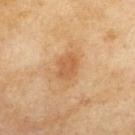Captured during whole-body skin photography for melanoma surveillance; the lesion was not biopsied.
A female subject in their 60s.
The lesion is located on the back.
Automated image analysis of the tile measured an area of roughly 6.5 mm², an outline eccentricity of about 0.65 (0 = round, 1 = elongated), and a shape-asymmetry score of about 0.2 (0 = symmetric). And it measured a mean CIELAB color near L≈53 a*≈20 b*≈37, roughly 8 lightness units darker than nearby skin, and a normalized border contrast of about 6. And it measured a border-irregularity rating of about 2/10. And it measured an automated nevus-likeness rating near 30 out of 100.
A roughly 15 mm field-of-view crop from a total-body skin photograph.
About 3.5 mm across.